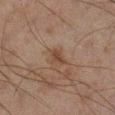workup: no biopsy performed (imaged during a skin exam)
imaging modality: ~15 mm crop, total-body skin-cancer survey
size: about 2.5 mm
patient: male, aged 43–47
body site: the right lower leg
automated lesion analysis: an average lesion color of about L≈35 a*≈15 b*≈24 (CIELAB), a lesion–skin lightness drop of about 6, and a lesion-to-skin contrast of about 6.5 (normalized; higher = more distinct); a detector confidence of about 100 out of 100 that the crop contains a lesion